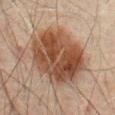No biopsy was performed on this lesion — it was imaged during a full skin examination and was not determined to be concerning.